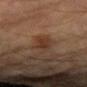* notes: imaged on a skin check; not biopsied
* anatomic site: the arm
* subject: male, in their mid- to late 80s or older
* image: ~15 mm tile from a whole-body skin photo